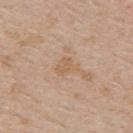This lesion was catalogued during total-body skin photography and was not selected for biopsy. This image is a 15 mm lesion crop taken from a total-body photograph. The tile uses white-light illumination. The lesion is located on the upper back. Measured at roughly 2.5 mm in maximum diameter. A male patient roughly 65 years of age.